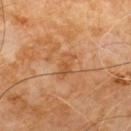| field | value |
|---|---|
| workup | no biopsy performed (imaged during a skin exam) |
| subject | male, aged approximately 60 |
| TBP lesion metrics | a lesion color around L≈52 a*≈23 b*≈38 in CIELAB, about 6 CIELAB-L* units darker than the surrounding skin, and a normalized border contrast of about 5.5; a within-lesion color-variation index near 3.5/10 |
| site | the chest |
| illumination | cross-polarized illumination |
| imaging modality | total-body-photography crop, ~15 mm field of view |